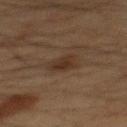follow-up: no biopsy performed (imaged during a skin exam) | anatomic site: the mid back | image-analysis metrics: a lesion color around L≈25 a*≈13 b*≈22 in CIELAB and a normalized lesion–skin contrast near 7.5; a detector confidence of about 100 out of 100 that the crop contains a lesion | lighting: cross-polarized | imaging modality: 15 mm crop, total-body photography | subject: male, aged approximately 65.